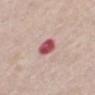No biopsy was performed on this lesion — it was imaged during a full skin examination and was not determined to be concerning. A 15 mm close-up extracted from a 3D total-body photography capture. The lesion-visualizer software estimated a lesion area of about 6 mm², an eccentricity of roughly 0.65, and a symmetry-axis asymmetry near 0.15. The analysis additionally found a lesion color around L≈53 a*≈32 b*≈21 in CIELAB and a lesion–skin lightness drop of about 18. The analysis additionally found border irregularity of about 1.5 on a 0–10 scale, internal color variation of about 4 on a 0–10 scale, and radial color variation of about 1.5. The subject is a male aged around 60. About 3 mm across. From the mid back. The tile uses white-light illumination.